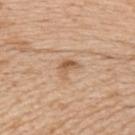The lesion was photographed on a routine skin check and not biopsied; there is no pathology result. The tile uses white-light illumination. A close-up tile cropped from a whole-body skin photograph, about 15 mm across. Longest diameter approximately 2.5 mm. The subject is a male aged 63–67. The lesion is located on the left upper arm. Automated tile analysis of the lesion measured a lesion area of about 3.5 mm², an outline eccentricity of about 0.75 (0 = round, 1 = elongated), and a symmetry-axis asymmetry near 0.45. And it measured a border-irregularity rating of about 4.5/10, internal color variation of about 3.5 on a 0–10 scale, and radial color variation of about 1. The analysis additionally found lesion-presence confidence of about 100/100.